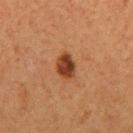About 3 mm across.
A region of skin cropped from a whole-body photographic capture, roughly 15 mm wide.
The lesion-visualizer software estimated a border-irregularity rating of about 2/10, a within-lesion color-variation index near 3.5/10, and peripheral color asymmetry of about 1.5. It also reported an automated nevus-likeness rating near 95 out of 100 and lesion-presence confidence of about 100/100.
The subject is a female about 40 years old.
Located on the right upper arm.
Imaged with cross-polarized lighting.